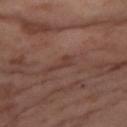acquisition: ~15 mm tile from a whole-body skin photo
site: the right thigh
patient: female, aged 53–57